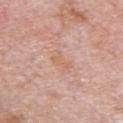Impression: Captured during whole-body skin photography for melanoma surveillance; the lesion was not biopsied. Context: Captured under white-light illumination. A 15 mm close-up extracted from a 3D total-body photography capture. On the chest. About 2.5 mm across. A male subject roughly 55 years of age.